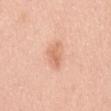notes = total-body-photography surveillance lesion; no biopsy
diameter = about 3.5 mm
image-analysis metrics = a footprint of about 4 mm²; an average lesion color of about L≈67 a*≈25 b*≈34 (CIELAB), roughly 10 lightness units darker than nearby skin, and a normalized border contrast of about 6; a border-irregularity rating of about 4.5/10, a color-variation rating of about 2.5/10, and peripheral color asymmetry of about 1; a nevus-likeness score of about 45/100 and lesion-presence confidence of about 100/100
tile lighting = white-light
subject = male, about 50 years old
site = the mid back
imaging modality = 15 mm crop, total-body photography